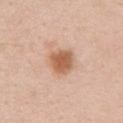Measured at roughly 3 mm in maximum diameter. Automated tile analysis of the lesion measured a lesion area of about 8 mm² and an eccentricity of roughly 0.3. The software also gave a border-irregularity index near 1.5/10 and a within-lesion color-variation index near 3/10. The lesion is located on the left upper arm. This image is a 15 mm lesion crop taken from a total-body photograph. Imaged with white-light lighting. A female patient, aged around 35.